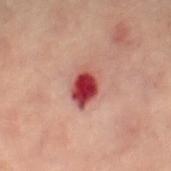Captured during whole-body skin photography for melanoma surveillance; the lesion was not biopsied. This image is a 15 mm lesion crop taken from a total-body photograph. Approximately 4.5 mm at its widest. A female subject aged around 60. The tile uses cross-polarized illumination. The lesion-visualizer software estimated a footprint of about 9 mm². And it measured a mean CIELAB color near L≈41 a*≈31 b*≈23, about 15 CIELAB-L* units darker than the surrounding skin, and a normalized border contrast of about 11.5. It also reported a nevus-likeness score of about 0/100 and lesion-presence confidence of about 100/100. From the left leg.